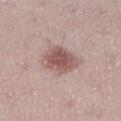workup — imaged on a skin check; not biopsied
site — the right lower leg
image — ~15 mm crop, total-body skin-cancer survey
patient — female, in their mid-20s